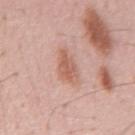Q: What is the lesion's diameter?
A: ≈4.5 mm
Q: Automated lesion metrics?
A: a border-irregularity rating of about 3.5/10 and a peripheral color-asymmetry measure near 1; a nevus-likeness score of about 0/100 and lesion-presence confidence of about 100/100
Q: Who is the patient?
A: male, about 55 years old
Q: How was this image acquired?
A: total-body-photography crop, ~15 mm field of view